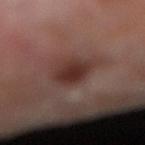{"biopsy_status": "not biopsied; imaged during a skin examination", "image": {"source": "total-body photography crop", "field_of_view_mm": 15}, "lesion_size": {"long_diameter_mm_approx": 4.0}, "site": "right lower leg", "patient": {"sex": "male", "age_approx": 70}}